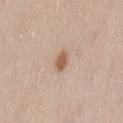Notes:
• biopsy status: catalogued during a skin exam; not biopsied
• image source: total-body-photography crop, ~15 mm field of view
• patient: female, aged around 40
• location: the lower back